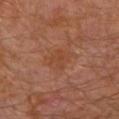biopsy status — catalogued during a skin exam; not biopsied
subject — male, aged 28–32
body site — the arm
imaging modality — ~15 mm crop, total-body skin-cancer survey